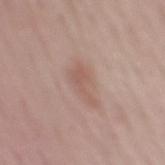This lesion was catalogued during total-body skin photography and was not selected for biopsy. Imaged with white-light lighting. A 15 mm close-up extracted from a 3D total-body photography capture. A male patient, aged 53–57. The lesion is on the mid back. Measured at roughly 4.5 mm in maximum diameter. The total-body-photography lesion software estimated a footprint of about 4.5 mm², an outline eccentricity of about 0.95 (0 = round, 1 = elongated), and a symmetry-axis asymmetry near 0.55. The analysis additionally found a border-irregularity index near 6.5/10, internal color variation of about 1.5 on a 0–10 scale, and radial color variation of about 0.5. And it measured a classifier nevus-likeness of about 5/100.